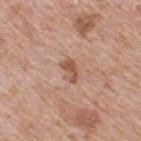Context:
Captured under white-light illumination. A male patient approximately 65 years of age. A 15 mm close-up tile from a total-body photography series done for melanoma screening. On the upper back. Longest diameter approximately 3 mm. The lesion-visualizer software estimated a mean CIELAB color near L≈54 a*≈22 b*≈31, about 10 CIELAB-L* units darker than the surrounding skin, and a lesion-to-skin contrast of about 7.5 (normalized; higher = more distinct). The software also gave a border-irregularity index near 3/10, internal color variation of about 3.5 on a 0–10 scale, and a peripheral color-asymmetry measure near 1. The analysis additionally found a detector confidence of about 100 out of 100 that the crop contains a lesion.